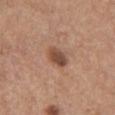This lesion was catalogued during total-body skin photography and was not selected for biopsy. A female subject, aged approximately 55. An algorithmic analysis of the crop reported an average lesion color of about L≈48 a*≈20 b*≈29 (CIELAB), about 12 CIELAB-L* units darker than the surrounding skin, and a lesion-to-skin contrast of about 8.5 (normalized; higher = more distinct). It also reported border irregularity of about 1.5 on a 0–10 scale. The software also gave an automated nevus-likeness rating near 75 out of 100. About 3 mm across. A 15 mm close-up extracted from a 3D total-body photography capture. From the chest. This is a white-light tile.